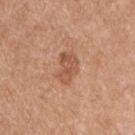Imaged during a routine full-body skin examination; the lesion was not biopsied and no histopathology is available. The tile uses white-light illumination. The patient is a male aged 53–57. On the front of the torso. A region of skin cropped from a whole-body photographic capture, roughly 15 mm wide. Automated image analysis of the tile measured a nevus-likeness score of about 20/100 and lesion-presence confidence of about 100/100. Measured at roughly 4 mm in maximum diameter.